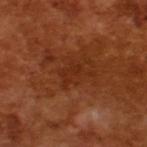A close-up tile cropped from a whole-body skin photograph, about 15 mm across. A male patient, aged approximately 65. The tile uses cross-polarized illumination. An algorithmic analysis of the crop reported a lesion area of about 5.5 mm² and an eccentricity of roughly 0.8. The software also gave roughly 6 lightness units darker than nearby skin and a lesion-to-skin contrast of about 5.5 (normalized; higher = more distinct). The software also gave a within-lesion color-variation index near 1.5/10 and radial color variation of about 0.5.